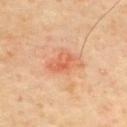The lesion was photographed on a routine skin check and not biopsied; there is no pathology result.
The recorded lesion diameter is about 4 mm.
The total-body-photography lesion software estimated an area of roughly 7.5 mm², an eccentricity of roughly 0.8, and a shape-asymmetry score of about 0.25 (0 = symmetric). The analysis additionally found a mean CIELAB color near L≈64 a*≈29 b*≈38 and roughly 9 lightness units darker than nearby skin. And it measured a border-irregularity index near 3/10 and peripheral color asymmetry of about 1.5. It also reported a classifier nevus-likeness of about 75/100 and lesion-presence confidence of about 100/100.
From the back.
The tile uses cross-polarized illumination.
A lesion tile, about 15 mm wide, cut from a 3D total-body photograph.
The patient is a male about 65 years old.